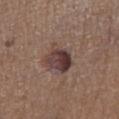Imaged with white-light lighting. A male subject, aged 63–67. A 15 mm close-up tile from a total-body photography series done for melanoma screening. On the right lower leg. The lesion-visualizer software estimated a lesion area of about 9.5 mm², an outline eccentricity of about 0.65 (0 = round, 1 = elongated), and a shape-asymmetry score of about 0.25 (0 = symmetric). The recorded lesion diameter is about 4 mm.